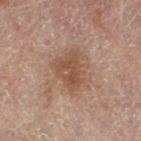Background: A roughly 15 mm field-of-view crop from a total-body skin photograph. A female patient, aged 78 to 82. The total-body-photography lesion software estimated an area of roughly 11 mm², an outline eccentricity of about 0.7 (0 = round, 1 = elongated), and a symmetry-axis asymmetry near 0.3. On the leg. Approximately 4.5 mm at its widest. Captured under cross-polarized illumination.